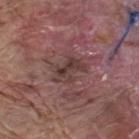Notes:
• biopsy status: imaged on a skin check; not biopsied
• image: ~15 mm tile from a whole-body skin photo
• size: about 4 mm
• location: the upper back
• patient: male, roughly 70 years of age